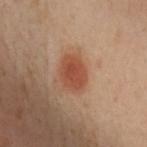biopsy status: total-body-photography surveillance lesion; no biopsy
image source: total-body-photography crop, ~15 mm field of view
subject: female, roughly 40 years of age
body site: the left upper arm
lighting: cross-polarized illumination
TBP lesion metrics: an area of roughly 8.5 mm² and an eccentricity of roughly 0.7; a lesion color around L≈49 a*≈26 b*≈33 in CIELAB, roughly 10 lightness units darker than nearby skin, and a normalized lesion–skin contrast near 8; internal color variation of about 2.5 on a 0–10 scale and radial color variation of about 1; an automated nevus-likeness rating near 100 out of 100 and a detector confidence of about 100 out of 100 that the crop contains a lesion